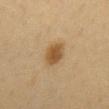Q: Is there a histopathology result?
A: imaged on a skin check; not biopsied
Q: What lighting was used for the tile?
A: cross-polarized illumination
Q: What is the lesion's diameter?
A: about 3 mm
Q: Lesion location?
A: the front of the torso
Q: What did automated image analysis measure?
A: a footprint of about 6.5 mm², an outline eccentricity of about 0.65 (0 = round, 1 = elongated), and a shape-asymmetry score of about 0.2 (0 = symmetric); a mean CIELAB color near L≈44 a*≈15 b*≈33, a lesion–skin lightness drop of about 10, and a normalized lesion–skin contrast near 8.5
Q: What are the patient's age and sex?
A: female, in their 40s
Q: What is the imaging modality?
A: 15 mm crop, total-body photography